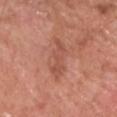Clinical impression:
The lesion was tiled from a total-body skin photograph and was not biopsied.
Image and clinical context:
The tile uses white-light illumination. Automated image analysis of the tile measured a footprint of about 9 mm² and an eccentricity of roughly 0.8. The analysis additionally found a mean CIELAB color near L≈53 a*≈25 b*≈30, a lesion–skin lightness drop of about 7, and a normalized border contrast of about 5. The software also gave internal color variation of about 2.5 on a 0–10 scale. A male patient aged around 80. From the leg. This image is a 15 mm lesion crop taken from a total-body photograph.